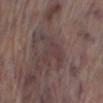biopsy status: total-body-photography surveillance lesion; no biopsy
patient: male, approximately 70 years of age
acquisition: 15 mm crop, total-body photography
location: the right lower leg
size: about 5 mm